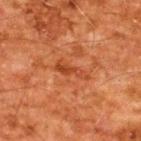Notes:
– biopsy status: no biopsy performed (imaged during a skin exam)
– patient: male, aged 58 to 62
– imaging modality: 15 mm crop, total-body photography
– site: the upper back
– lesion diameter: ~4 mm (longest diameter)